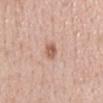biopsy status: imaged on a skin check; not biopsied | TBP lesion metrics: an area of roughly 3 mm², a shape eccentricity near 0.9, and a shape-asymmetry score of about 0.25 (0 = symmetric); a border-irregularity rating of about 2.5/10, internal color variation of about 3 on a 0–10 scale, and a peripheral color-asymmetry measure near 1.5; an automated nevus-likeness rating near 90 out of 100 and lesion-presence confidence of about 100/100 | lesion size: ≈3 mm | site: the back | imaging modality: total-body-photography crop, ~15 mm field of view | subject: male, roughly 50 years of age.